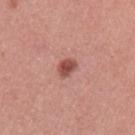No biopsy was performed on this lesion — it was imaged during a full skin examination and was not determined to be concerning. This is a white-light tile. The lesion's longest dimension is about 2.5 mm. The total-body-photography lesion software estimated a footprint of about 4 mm², an eccentricity of roughly 0.7, and a symmetry-axis asymmetry near 0.25. A female subject in their mid- to late 30s. The lesion is located on the left upper arm. A 15 mm close-up extracted from a 3D total-body photography capture.